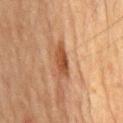Findings:
– notes: total-body-photography surveillance lesion; no biopsy
– body site: the back
– image-analysis metrics: a footprint of about 7 mm², a shape eccentricity near 0.9, and a shape-asymmetry score of about 0.25 (0 = symmetric); an automated nevus-likeness rating near 75 out of 100 and a detector confidence of about 100 out of 100 that the crop contains a lesion
– size: ≈4.5 mm
– subject: male, in their 70s
– image: 15 mm crop, total-body photography